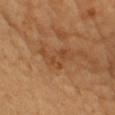Impression:
Recorded during total-body skin imaging; not selected for excision or biopsy.
Background:
Captured under cross-polarized illumination. This image is a 15 mm lesion crop taken from a total-body photograph. A female patient aged approximately 55. Located on the chest. The lesion-visualizer software estimated a mean CIELAB color near L≈47 a*≈23 b*≈37, about 7 CIELAB-L* units darker than the surrounding skin, and a lesion-to-skin contrast of about 5.5 (normalized; higher = more distinct). The software also gave a nevus-likeness score of about 0/100 and a lesion-detection confidence of about 100/100.